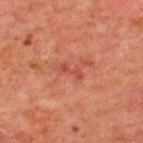  biopsy_status: not biopsied; imaged during a skin examination
  image:
    source: total-body photography crop
    field_of_view_mm: 15
  automated_metrics:
    area_mm2_approx: 2.5
    eccentricity: 0.95
    shape_asymmetry: 0.35
    color_variation_0_10: 0.0
    peripheral_color_asymmetry: 0.0
  site: upper back
  patient:
    sex: male
    age_approx: 60
  lighting: cross-polarized
  lesion_size:
    long_diameter_mm_approx: 3.0